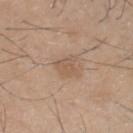Impression:
Recorded during total-body skin imaging; not selected for excision or biopsy.
Acquisition and patient details:
A male subject, aged around 45. On the head or neck. A 15 mm crop from a total-body photograph taken for skin-cancer surveillance. Measured at roughly 2.5 mm in maximum diameter. An algorithmic analysis of the crop reported a footprint of about 4.5 mm², an eccentricity of roughly 0.4, and a symmetry-axis asymmetry near 0.3. The analysis additionally found a lesion color around L≈56 a*≈17 b*≈30 in CIELAB, roughly 7 lightness units darker than nearby skin, and a normalized lesion–skin contrast near 5.